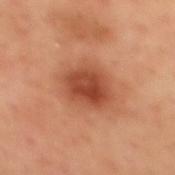workup = total-body-photography surveillance lesion; no biopsy | subject = female, in their 50s | size = about 4.5 mm | TBP lesion metrics = roughly 13 lightness units darker than nearby skin and a normalized lesion–skin contrast near 9; a nevus-likeness score of about 100/100 | tile lighting = cross-polarized illumination | image = ~15 mm tile from a whole-body skin photo | body site = the mid back.